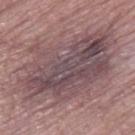notes = total-body-photography surveillance lesion; no biopsy | anatomic site = the right thigh | patient = female, aged 68 to 72 | imaging modality = 15 mm crop, total-body photography.